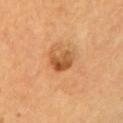<case>
<biopsy_status>not biopsied; imaged during a skin examination</biopsy_status>
<patient>
  <sex>male</sex>
  <age_approx>55</age_approx>
</patient>
<site>left upper arm</site>
<image>
  <source>total-body photography crop</source>
  <field_of_view_mm>15</field_of_view_mm>
</image>
</case>